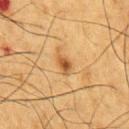{"biopsy_status": "not biopsied; imaged during a skin examination", "site": "chest", "patient": {"sex": "male", "age_approx": 55}, "image": {"source": "total-body photography crop", "field_of_view_mm": 15}}